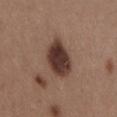notes: imaged on a skin check; not biopsied
TBP lesion metrics: an area of roughly 14 mm², an eccentricity of roughly 0.8, and a shape-asymmetry score of about 0.15 (0 = symmetric); lesion-presence confidence of about 100/100
anatomic site: the lower back
subject: female, in their 30s
imaging modality: 15 mm crop, total-body photography